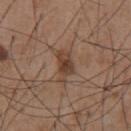Assessment:
Captured during whole-body skin photography for melanoma surveillance; the lesion was not biopsied.
Context:
A close-up tile cropped from a whole-body skin photograph, about 15 mm across. A male subject, approximately 55 years of age. The lesion is on the abdomen.